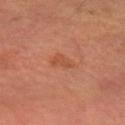Part of a total-body skin-imaging series; this lesion was reviewed on a skin check and was not flagged for biopsy.
A roughly 15 mm field-of-view crop from a total-body skin photograph.
The lesion is on the left forearm.
The recorded lesion diameter is about 3 mm.
The patient is a male aged 58 to 62.